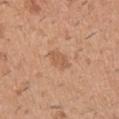Assessment:
Captured during whole-body skin photography for melanoma surveillance; the lesion was not biopsied.
Context:
This image is a 15 mm lesion crop taken from a total-body photograph. The lesion is on the right upper arm. The subject is a male about 40 years old.